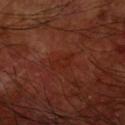Acquisition and patient details: A 15 mm close-up extracted from a 3D total-body photography capture. A male patient approximately 60 years of age. Approximately 3 mm at its widest. Automated tile analysis of the lesion measured a lesion color around L≈25 a*≈26 b*≈28 in CIELAB and a lesion-to-skin contrast of about 5 (normalized; higher = more distinct). The analysis additionally found a border-irregularity rating of about 4/10, a within-lesion color-variation index near 1.5/10, and a peripheral color-asymmetry measure near 0.5. The software also gave a nevus-likeness score of about 0/100 and a lesion-detection confidence of about 95/100. The lesion is located on the right upper arm.